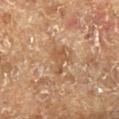Imaged during a routine full-body skin examination; the lesion was not biopsied and no histopathology is available.
The lesion is on the right lower leg.
A 15 mm crop from a total-body photograph taken for skin-cancer surveillance.
A male subject, aged around 75.
The lesion's longest dimension is about 3 mm.
Captured under cross-polarized illumination.